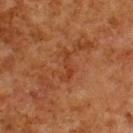Background:
A male patient, about 80 years old. Cropped from a total-body skin-imaging series; the visible field is about 15 mm. The lesion-visualizer software estimated a mean CIELAB color near L≈31 a*≈23 b*≈30, a lesion–skin lightness drop of about 5, and a normalized border contrast of about 5.5. And it measured border irregularity of about 7.5 on a 0–10 scale, internal color variation of about 0 on a 0–10 scale, and radial color variation of about 0. The software also gave a classifier nevus-likeness of about 0/100 and a detector confidence of about 100 out of 100 that the crop contains a lesion. On the upper back.